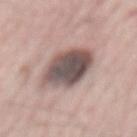Clinical impression: Part of a total-body skin-imaging series; this lesion was reviewed on a skin check and was not flagged for biopsy. Background: The lesion is on the mid back. A 15 mm close-up tile from a total-body photography series done for melanoma screening. A male patient aged approximately 65.